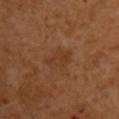notes: no biopsy performed (imaged during a skin exam).